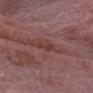| key | value |
|---|---|
| biopsy status | catalogued during a skin exam; not biopsied |
| diameter | ~2.5 mm (longest diameter) |
| patient | male, in their mid- to late 60s |
| image | 15 mm crop, total-body photography |
| illumination | white-light illumination |
| site | the head or neck |